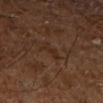Impression: No biopsy was performed on this lesion — it was imaged during a full skin examination and was not determined to be concerning. Image and clinical context: A male subject roughly 60 years of age. The recorded lesion diameter is about 2.5 mm. Imaged with cross-polarized lighting. A lesion tile, about 15 mm wide, cut from a 3D total-body photograph. On the right leg. The lesion-visualizer software estimated a lesion area of about 2.5 mm² and an eccentricity of roughly 0.9. The software also gave a border-irregularity rating of about 6/10. And it measured lesion-presence confidence of about 95/100.